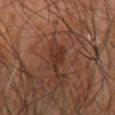{
  "automated_metrics": {
    "area_mm2_approx": 4.5,
    "eccentricity": 0.6,
    "shape_asymmetry": 0.35,
    "color_variation_0_10": 2.0,
    "peripheral_color_asymmetry": 1.0
  },
  "lesion_size": {
    "long_diameter_mm_approx": 3.0
  },
  "site": "left upper arm",
  "patient": {
    "sex": "male",
    "age_approx": 65
  },
  "lighting": "cross-polarized",
  "image": {
    "source": "total-body photography crop",
    "field_of_view_mm": 15
  }
}